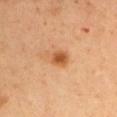Part of a total-body skin-imaging series; this lesion was reviewed on a skin check and was not flagged for biopsy. Measured at roughly 3 mm in maximum diameter. A 15 mm crop from a total-body photograph taken for skin-cancer surveillance. The lesion is located on the left upper arm. The patient is a male aged 53–57.